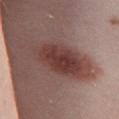Q: Was this lesion biopsied?
A: imaged on a skin check; not biopsied
Q: What is the lesion's diameter?
A: ~7 mm (longest diameter)
Q: What lighting was used for the tile?
A: white-light illumination
Q: What is the anatomic site?
A: the abdomen
Q: Who is the patient?
A: female, approximately 25 years of age
Q: What kind of image is this?
A: 15 mm crop, total-body photography
Q: Automated lesion metrics?
A: a footprint of about 20 mm² and an eccentricity of roughly 0.85; a lesion color around L≈39 a*≈22 b*≈22 in CIELAB and a lesion-to-skin contrast of about 9.5 (normalized; higher = more distinct); an automated nevus-likeness rating near 100 out of 100